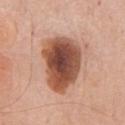Imaged during a routine full-body skin examination; the lesion was not biopsied and no histopathology is available.
A male subject aged 78 to 82.
Cropped from a whole-body photographic skin survey; the tile spans about 15 mm.
An algorithmic analysis of the crop reported internal color variation of about 8 on a 0–10 scale and a peripheral color-asymmetry measure near 2. The software also gave a nevus-likeness score of about 80/100 and a lesion-detection confidence of about 100/100.
The tile uses white-light illumination.
From the chest.
The recorded lesion diameter is about 7 mm.